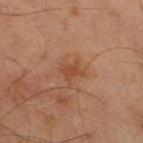Clinical summary:
Captured under cross-polarized illumination. This image is a 15 mm lesion crop taken from a total-body photograph. From the right thigh. A male patient roughly 60 years of age. Measured at roughly 2.5 mm in maximum diameter. Automated tile analysis of the lesion measured a mean CIELAB color near L≈42 a*≈22 b*≈30 and a normalized border contrast of about 6. The analysis additionally found a border-irregularity rating of about 4/10 and radial color variation of about 0.5.